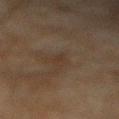workup=total-body-photography surveillance lesion; no biopsy
site=the arm
illumination=cross-polarized illumination
lesion diameter=about 2.5 mm
patient=male, aged approximately 45
automated metrics=a footprint of about 3 mm² and two-axis asymmetry of about 0.35; an average lesion color of about L≈27 a*≈11 b*≈21 (CIELAB) and a normalized lesion–skin contrast near 5; a nevus-likeness score of about 0/100
imaging modality=total-body-photography crop, ~15 mm field of view